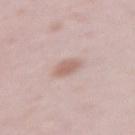| field | value |
|---|---|
| biopsy status | catalogued during a skin exam; not biopsied |
| acquisition | ~15 mm crop, total-body skin-cancer survey |
| illumination | white-light illumination |
| automated lesion analysis | a footprint of about 4.5 mm², an outline eccentricity of about 0.7 (0 = round, 1 = elongated), and a shape-asymmetry score of about 0.15 (0 = symmetric); a mean CIELAB color near L≈62 a*≈18 b*≈24, about 10 CIELAB-L* units darker than the surrounding skin, and a normalized lesion–skin contrast near 6.5 |
| subject | female, about 15 years old |
| location | the left upper arm |
| size | ~3 mm (longest diameter) |